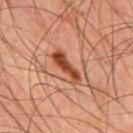Recorded during total-body skin imaging; not selected for excision or biopsy. A 15 mm close-up tile from a total-body photography series done for melanoma screening. The tile uses cross-polarized illumination. Located on the mid back. The patient is a male approximately 45 years of age.